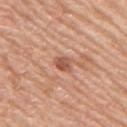– follow-up: total-body-photography surveillance lesion; no biopsy
– lesion diameter: ≈2.5 mm
– anatomic site: the back
– image-analysis metrics: an area of roughly 3.5 mm², an outline eccentricity of about 0.7 (0 = round, 1 = elongated), and a symmetry-axis asymmetry near 0.25; an average lesion color of about L≈55 a*≈25 b*≈32 (CIELAB), about 11 CIELAB-L* units darker than the surrounding skin, and a lesion-to-skin contrast of about 7.5 (normalized; higher = more distinct)
– subject: male, aged approximately 60
– imaging modality: total-body-photography crop, ~15 mm field of view